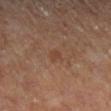Impression:
The lesion was photographed on a routine skin check and not biopsied; there is no pathology result.
Acquisition and patient details:
Captured under cross-polarized illumination. Located on the right lower leg. A male subject aged 58–62. The lesion's longest dimension is about 2.5 mm. A region of skin cropped from a whole-body photographic capture, roughly 15 mm wide.